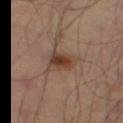This lesion was catalogued during total-body skin photography and was not selected for biopsy. Automated tile analysis of the lesion measured a mean CIELAB color near L≈39 a*≈18 b*≈27, about 10 CIELAB-L* units darker than the surrounding skin, and a lesion-to-skin contrast of about 9 (normalized; higher = more distinct). The analysis additionally found a border-irregularity rating of about 2.5/10 and a peripheral color-asymmetry measure near 1.5. A lesion tile, about 15 mm wide, cut from a 3D total-body photograph. The lesion is on the leg. The lesion's longest dimension is about 3.5 mm. This is a cross-polarized tile. A male subject, aged 48 to 52.